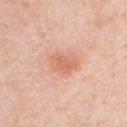Impression:
The lesion was tiled from a total-body skin photograph and was not biopsied.
Acquisition and patient details:
A male subject aged approximately 40. Located on the chest. About 4 mm across. The tile uses white-light illumination. Cropped from a whole-body photographic skin survey; the tile spans about 15 mm.